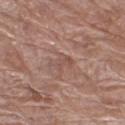The lesion was photographed on a routine skin check and not biopsied; there is no pathology result.
Imaged with white-light lighting.
Located on the right thigh.
Cropped from a whole-body photographic skin survey; the tile spans about 15 mm.
A female subject, approximately 70 years of age.